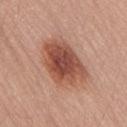Impression:
Captured during whole-body skin photography for melanoma surveillance; the lesion was not biopsied.
Acquisition and patient details:
Captured under white-light illumination. The lesion is on the lower back. The subject is a male aged 63–67. Cropped from a total-body skin-imaging series; the visible field is about 15 mm.